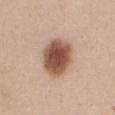{"biopsy_status": "not biopsied; imaged during a skin examination"}